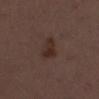notes: catalogued during a skin exam; not biopsied | site: the right lower leg | image source: total-body-photography crop, ~15 mm field of view | patient: female, aged 48–52 | automated lesion analysis: a lesion area of about 6 mm², an eccentricity of roughly 0.75, and a symmetry-axis asymmetry near 0.3; roughly 7 lightness units darker than nearby skin and a normalized border contrast of about 7.5; a classifier nevus-likeness of about 65/100 and a lesion-detection confidence of about 100/100 | diameter: ~3 mm (longest diameter) | illumination: white-light.